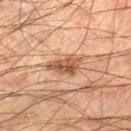Notes:
– notes — total-body-photography surveillance lesion; no biopsy
– subject — male, aged 43–47
– acquisition — ~15 mm tile from a whole-body skin photo
– size — about 4.5 mm
– image-analysis metrics — a mean CIELAB color near L≈52 a*≈22 b*≈33, a lesion–skin lightness drop of about 12, and a normalized border contrast of about 8; an automated nevus-likeness rating near 75 out of 100
– site — the right lower leg